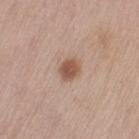This lesion was catalogued during total-body skin photography and was not selected for biopsy. This is a white-light tile. A female patient, approximately 65 years of age. Longest diameter approximately 2.5 mm. Located on the left thigh. A 15 mm close-up extracted from a 3D total-body photography capture. Automated tile analysis of the lesion measured a footprint of about 5 mm², an eccentricity of roughly 0.55, and a symmetry-axis asymmetry near 0.3. The analysis additionally found a within-lesion color-variation index near 2.5/10 and a peripheral color-asymmetry measure near 1.